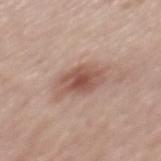Findings:
- notes — imaged on a skin check; not biopsied
- tile lighting — white-light
- image — total-body-photography crop, ~15 mm field of view
- site — the mid back
- size — ≈4.5 mm
- patient — female, aged around 65